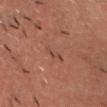No biopsy was performed on this lesion — it was imaged during a full skin examination and was not determined to be concerning. A male subject aged 58 to 62. Located on the chest. A roughly 15 mm field-of-view crop from a total-body skin photograph. The tile uses cross-polarized illumination.